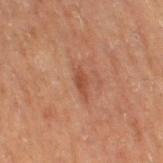Q: Is there a histopathology result?
A: catalogued during a skin exam; not biopsied
Q: What is the anatomic site?
A: the right thigh
Q: How was this image acquired?
A: total-body-photography crop, ~15 mm field of view
Q: Who is the patient?
A: male, aged around 70